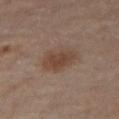No biopsy was performed on this lesion — it was imaged during a full skin examination and was not determined to be concerning. The lesion is located on the right thigh. The patient is a female aged approximately 50. A 15 mm close-up tile from a total-body photography series done for melanoma screening.